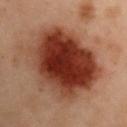workup: catalogued during a skin exam; not biopsied
image-analysis metrics: a lesion color around L≈29 a*≈23 b*≈26 in CIELAB, about 15 CIELAB-L* units darker than the surrounding skin, and a lesion-to-skin contrast of about 14 (normalized; higher = more distinct); a border-irregularity rating of about 1.5/10, a color-variation rating of about 7/10, and peripheral color asymmetry of about 2; a classifier nevus-likeness of about 100/100
patient: male, in their mid- to late 50s
site: the arm
lesion diameter: ~9 mm (longest diameter)
image source: ~15 mm tile from a whole-body skin photo